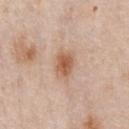{
  "site": "chest",
  "lighting": "white-light",
  "image": {
    "source": "total-body photography crop",
    "field_of_view_mm": 15
  },
  "patient": {
    "sex": "male",
    "age_approx": 60
  }
}